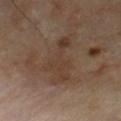illumination: cross-polarized; size: about 6.5 mm; image source: ~15 mm crop, total-body skin-cancer survey; location: the chest; patient: male, aged around 70.